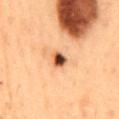Clinical impression:
Recorded during total-body skin imaging; not selected for excision or biopsy.
Acquisition and patient details:
Approximately 2.5 mm at its widest. A male patient, roughly 55 years of age. Automated tile analysis of the lesion measured about 20 CIELAB-L* units darker than the surrounding skin and a lesion-to-skin contrast of about 14 (normalized; higher = more distinct). It also reported a classifier nevus-likeness of about 100/100 and a lesion-detection confidence of about 100/100. Captured under cross-polarized illumination. The lesion is on the mid back. A roughly 15 mm field-of-view crop from a total-body skin photograph.